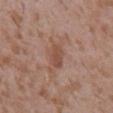Notes:
* workup: imaged on a skin check; not biopsied
* lesion size: ~3.5 mm (longest diameter)
* site: the back
* lighting: white-light illumination
* image source: total-body-photography crop, ~15 mm field of view
* patient: male, approximately 45 years of age